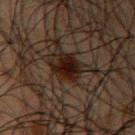This lesion was catalogued during total-body skin photography and was not selected for biopsy.